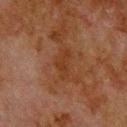Recorded during total-body skin imaging; not selected for excision or biopsy. A 15 mm close-up tile from a total-body photography series done for melanoma screening. From the upper back. A male subject, about 80 years old.